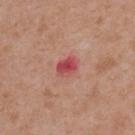{"biopsy_status": "not biopsied; imaged during a skin examination", "lesion_size": {"long_diameter_mm_approx": 2.5}, "image": {"source": "total-body photography crop", "field_of_view_mm": 15}, "automated_metrics": {"cielab_L": 50, "cielab_a": 36, "cielab_b": 24, "nevus_likeness_0_100": 0, "lesion_detection_confidence_0_100": 100}, "site": "upper back", "lighting": "white-light", "patient": {"sex": "female", "age_approx": 40}}